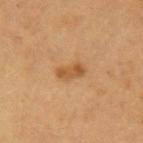Assessment: This lesion was catalogued during total-body skin photography and was not selected for biopsy. Clinical summary: This is a cross-polarized tile. Approximately 3 mm at its widest. Located on the left upper arm. Automated image analysis of the tile measured a lesion color around L≈45 a*≈19 b*≈35 in CIELAB, about 8 CIELAB-L* units darker than the surrounding skin, and a normalized lesion–skin contrast near 7. The analysis additionally found a color-variation rating of about 3/10 and radial color variation of about 1. It also reported a classifier nevus-likeness of about 50/100. A female subject roughly 40 years of age. A region of skin cropped from a whole-body photographic capture, roughly 15 mm wide.